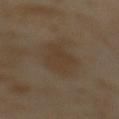{"biopsy_status": "not biopsied; imaged during a skin examination", "automated_metrics": {"color_variation_0_10": 2.0, "peripheral_color_asymmetry": 0.5, "nevus_likeness_0_100": 0, "lesion_detection_confidence_0_100": 100}, "lesion_size": {"long_diameter_mm_approx": 4.5}, "image": {"source": "total-body photography crop", "field_of_view_mm": 15}, "site": "mid back", "lighting": "cross-polarized", "patient": {"sex": "female", "age_approx": 55}}